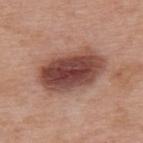notes = total-body-photography surveillance lesion; no biopsy | site = the upper back | image = ~15 mm crop, total-body skin-cancer survey | lighting = white-light illumination | subject = female, aged 38 to 42.